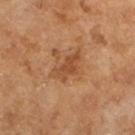Imaged during a routine full-body skin examination; the lesion was not biopsied and no histopathology is available. The total-body-photography lesion software estimated a lesion area of about 6.5 mm², a shape eccentricity near 0.85, and a shape-asymmetry score of about 0.4 (0 = symmetric). It also reported internal color variation of about 2 on a 0–10 scale. The tile uses cross-polarized illumination. The lesion is on the left lower leg. This image is a 15 mm lesion crop taken from a total-body photograph. The lesion's longest dimension is about 4 mm. A male subject, aged around 65.